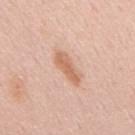Clinical impression: Captured during whole-body skin photography for melanoma surveillance; the lesion was not biopsied. Context: The patient is a male aged 53 to 57. A close-up tile cropped from a whole-body skin photograph, about 15 mm across. Located on the mid back.